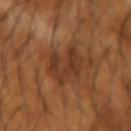Captured during whole-body skin photography for melanoma surveillance; the lesion was not biopsied. A male patient in their mid-60s. From the left forearm. A close-up tile cropped from a whole-body skin photograph, about 15 mm across.